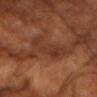The lesion was tiled from a total-body skin photograph and was not biopsied. A male patient, aged 58 to 62. Cropped from a whole-body photographic skin survey; the tile spans about 15 mm. This is a cross-polarized tile. From the chest.